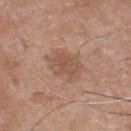  biopsy_status: not biopsied; imaged during a skin examination
  image:
    source: total-body photography crop
    field_of_view_mm: 15
  site: chest
  lesion_size:
    long_diameter_mm_approx: 4.5
  patient:
    sex: male
    age_approx: 75
  lighting: white-light